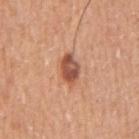biopsy status: no biopsy performed (imaged during a skin exam); size: about 3.5 mm; illumination: white-light; imaging modality: 15 mm crop, total-body photography; subject: male, aged approximately 55; body site: the right upper arm.